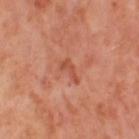| key | value |
|---|---|
| biopsy status | catalogued during a skin exam; not biopsied |
| subject | female, about 55 years old |
| location | the left thigh |
| acquisition | 15 mm crop, total-body photography |